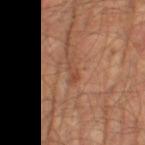Captured during whole-body skin photography for melanoma surveillance; the lesion was not biopsied. Imaged with cross-polarized lighting. About 3 mm across. This image is a 15 mm lesion crop taken from a total-body photograph. The lesion is located on the leg. A male patient, approximately 65 years of age. The total-body-photography lesion software estimated an average lesion color of about L≈46 a*≈23 b*≈31 (CIELAB) and a normalized lesion–skin contrast near 5.5. The software also gave an automated nevus-likeness rating near 0 out of 100.